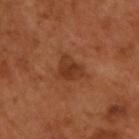Captured during whole-body skin photography for melanoma surveillance; the lesion was not biopsied. The tile uses cross-polarized illumination. Automated tile analysis of the lesion measured a lesion area of about 5.5 mm², an eccentricity of roughly 0.6, and two-axis asymmetry of about 0.4. A male patient approximately 50 years of age. On the upper back. The recorded lesion diameter is about 3 mm. A 15 mm crop from a total-body photograph taken for skin-cancer surveillance.